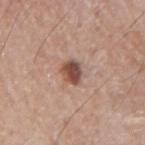{
  "biopsy_status": "not biopsied; imaged during a skin examination",
  "patient": {
    "sex": "male",
    "age_approx": 75
  },
  "lesion_size": {
    "long_diameter_mm_approx": 3.0
  },
  "automated_metrics": {
    "nevus_likeness_0_100": 95,
    "lesion_detection_confidence_0_100": 100
  },
  "lighting": "white-light",
  "site": "right upper arm",
  "image": {
    "source": "total-body photography crop",
    "field_of_view_mm": 15
  }
}